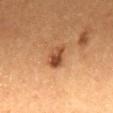{
  "image": {
    "source": "total-body photography crop",
    "field_of_view_mm": 15
  },
  "lighting": "cross-polarized",
  "automated_metrics": {
    "cielab_L": 40,
    "cielab_a": 21,
    "cielab_b": 31,
    "vs_skin_darker_L": 11.0,
    "vs_skin_contrast_norm": 9.0
  },
  "patient": {
    "sex": "female",
    "age_approx": 40
  },
  "site": "mid back",
  "lesion_size": {
    "long_diameter_mm_approx": 3.5
  }
}